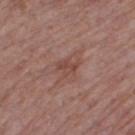notes: imaged on a skin check; not biopsied
size: ~2.5 mm (longest diameter)
image source: ~15 mm crop, total-body skin-cancer survey
subject: male, approximately 70 years of age
image-analysis metrics: a footprint of about 3.5 mm², an eccentricity of roughly 0.65, and a shape-asymmetry score of about 0.35 (0 = symmetric); about 7 CIELAB-L* units darker than the surrounding skin and a normalized border contrast of about 6; a border-irregularity index near 4/10, internal color variation of about 1.5 on a 0–10 scale, and a peripheral color-asymmetry measure near 0.5; lesion-presence confidence of about 100/100
site: the right thigh
lighting: white-light illumination